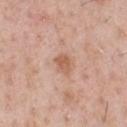Recorded during total-body skin imaging; not selected for excision or biopsy. The lesion is located on the chest. A roughly 15 mm field-of-view crop from a total-body skin photograph. The subject is a male about 50 years old.